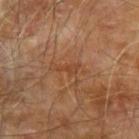Imaged during a routine full-body skin examination; the lesion was not biopsied and no histopathology is available. Approximately 2.5 mm at its widest. This is a cross-polarized tile. Located on the left forearm. The lesion-visualizer software estimated roughly 6 lightness units darker than nearby skin and a normalized border contrast of about 5. The analysis additionally found a classifier nevus-likeness of about 0/100 and a lesion-detection confidence of about 95/100. This image is a 15 mm lesion crop taken from a total-body photograph. The patient is a male approximately 70 years of age.